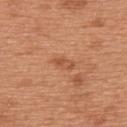No biopsy was performed on this lesion — it was imaged during a full skin examination and was not determined to be concerning. A roughly 15 mm field-of-view crop from a total-body skin photograph. The tile uses white-light illumination. A male patient, aged 63 to 67. The recorded lesion diameter is about 3 mm. The lesion is on the upper back.